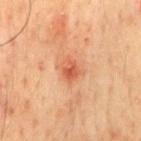Recorded during total-body skin imaging; not selected for excision or biopsy.
This image is a 15 mm lesion crop taken from a total-body photograph.
The subject is a male aged 48 to 52.
The lesion is located on the chest.